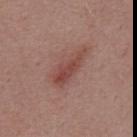Context: Longest diameter approximately 5.5 mm. The tile uses white-light illumination. The patient is a male aged approximately 55. Located on the back. This image is a 15 mm lesion crop taken from a total-body photograph. An algorithmic analysis of the crop reported about 9 CIELAB-L* units darker than the surrounding skin. The software also gave a classifier nevus-likeness of about 20/100 and lesion-presence confidence of about 100/100.